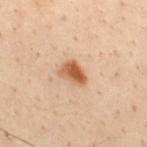  lighting: cross-polarized
  site: mid back
  patient:
    sex: male
    age_approx: 30
  image:
    source: total-body photography crop
    field_of_view_mm: 15